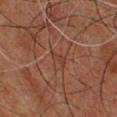Context: Cropped from a whole-body photographic skin survey; the tile spans about 15 mm. A male patient aged approximately 60. Longest diameter approximately 3 mm. This is a cross-polarized tile. From the chest.